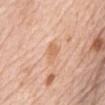notes = no biopsy performed (imaged during a skin exam) | illumination = white-light illumination | anatomic site = the chest | image-analysis metrics = an average lesion color of about L≈66 a*≈22 b*≈36 (CIELAB), a lesion–skin lightness drop of about 7, and a normalized lesion–skin contrast near 5.5; a border-irregularity rating of about 2.5/10 and a color-variation rating of about 1.5/10 | subject = male, aged 78 to 82 | diameter = about 2.5 mm | imaging modality = ~15 mm crop, total-body skin-cancer survey.